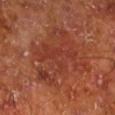Recorded during total-body skin imaging; not selected for excision or biopsy.
A 15 mm close-up tile from a total-body photography series done for melanoma screening.
From the left lower leg.
A male patient, about 65 years old.